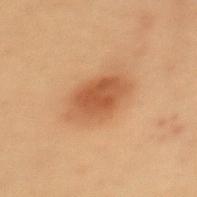Assessment:
No biopsy was performed on this lesion — it was imaged during a full skin examination and was not determined to be concerning.
Image and clinical context:
The lesion is on the upper back. A female patient, aged approximately 40. A roughly 15 mm field-of-view crop from a total-body skin photograph. The tile uses cross-polarized illumination. Measured at roughly 5.5 mm in maximum diameter.